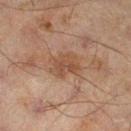Captured during whole-body skin photography for melanoma surveillance; the lesion was not biopsied. This is a cross-polarized tile. The subject is a male aged around 45. From the left lower leg. This image is a 15 mm lesion crop taken from a total-body photograph. The lesion's longest dimension is about 3.5 mm.